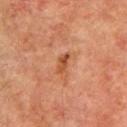The lesion was tiled from a total-body skin photograph and was not biopsied. A male patient, aged 73–77. Automated image analysis of the tile measured an area of roughly 3 mm², an outline eccentricity of about 0.9 (0 = round, 1 = elongated), and a symmetry-axis asymmetry near 0.45. It also reported an average lesion color of about L≈41 a*≈24 b*≈32 (CIELAB), roughly 9 lightness units darker than nearby skin, and a normalized lesion–skin contrast near 8. The software also gave a border-irregularity rating of about 4/10 and radial color variation of about 0. The lesion's longest dimension is about 2.5 mm. Captured under cross-polarized illumination. The lesion is on the left upper arm. Cropped from a total-body skin-imaging series; the visible field is about 15 mm.